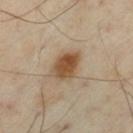Q: What kind of image is this?
A: ~15 mm crop, total-body skin-cancer survey
Q: How was the tile lit?
A: cross-polarized
Q: What is the lesion's diameter?
A: ~3.5 mm (longest diameter)
Q: What are the patient's age and sex?
A: male, approximately 55 years of age
Q: Where on the body is the lesion?
A: the left thigh